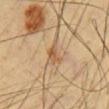No biopsy was performed on this lesion — it was imaged during a full skin examination and was not determined to be concerning.